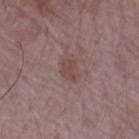biopsy status = no biopsy performed (imaged during a skin exam)
image = ~15 mm crop, total-body skin-cancer survey
subject = male, aged 63–67
automated metrics = a lesion color around L≈45 a*≈18 b*≈20 in CIELAB, a lesion–skin lightness drop of about 7, and a normalized border contrast of about 6; border irregularity of about 2.5 on a 0–10 scale, internal color variation of about 1.5 on a 0–10 scale, and peripheral color asymmetry of about 0.5; an automated nevus-likeness rating near 0 out of 100 and a lesion-detection confidence of about 100/100
illumination = white-light
lesion size = about 3 mm
site = the left forearm